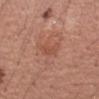The lesion was photographed on a routine skin check and not biopsied; there is no pathology result.
The lesion is on the left upper arm.
A roughly 15 mm field-of-view crop from a total-body skin photograph.
About 3.5 mm across.
The subject is a female aged 53 to 57.
The lesion-visualizer software estimated a border-irregularity index near 4/10. The software also gave a nevus-likeness score of about 60/100 and a detector confidence of about 100 out of 100 that the crop contains a lesion.
Captured under white-light illumination.